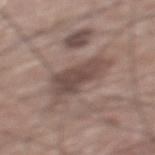On the mid back. The patient is a male roughly 60 years of age. The recorded lesion diameter is about 6 mm. Cropped from a whole-body photographic skin survey; the tile spans about 15 mm. Imaged with white-light lighting.